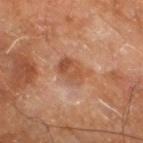follow-up — no biopsy performed (imaged during a skin exam); image source — total-body-photography crop, ~15 mm field of view; tile lighting — cross-polarized; diameter — ≈3.5 mm; anatomic site — the leg; subject — male, aged around 60.